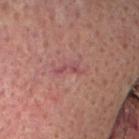lesion size: about 3.5 mm | acquisition: ~15 mm crop, total-body skin-cancer survey | patient: male, aged around 55 | illumination: cross-polarized | image-analysis metrics: a shape-asymmetry score of about 0.55 (0 = symmetric); an average lesion color of about L≈45 a*≈25 b*≈19 (CIELAB) and a normalized border contrast of about 6; border irregularity of about 7 on a 0–10 scale, a within-lesion color-variation index near 0/10, and a peripheral color-asymmetry measure near 0; a lesion-detection confidence of about 75/100 | site: the head or neck.